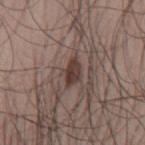This lesion was catalogued during total-body skin photography and was not selected for biopsy. A male patient aged 48–52. Automated tile analysis of the lesion measured a mean CIELAB color near L≈38 a*≈17 b*≈20, about 11 CIELAB-L* units darker than the surrounding skin, and a normalized border contrast of about 9.5. The software also gave lesion-presence confidence of about 100/100. A region of skin cropped from a whole-body photographic capture, roughly 15 mm wide. Captured under white-light illumination. About 3.5 mm across. On the mid back.